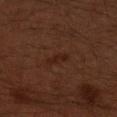Imaged during a routine full-body skin examination; the lesion was not biopsied and no histopathology is available. The total-body-photography lesion software estimated a footprint of about 2.5 mm², a shape eccentricity near 0.95, and a symmetry-axis asymmetry near 0.3. On the right upper arm. Imaged with cross-polarized lighting. A close-up tile cropped from a whole-body skin photograph, about 15 mm across. A male patient aged around 60. About 3 mm across.